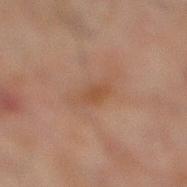The lesion was tiled from a total-body skin photograph and was not biopsied. The recorded lesion diameter is about 4.5 mm. Located on the right lower leg. A male patient, aged approximately 45. This is a cross-polarized tile. A region of skin cropped from a whole-body photographic capture, roughly 15 mm wide.